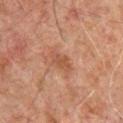  biopsy_status: not biopsied; imaged during a skin examination
  image:
    source: total-body photography crop
    field_of_view_mm: 15
  lesion_size:
    long_diameter_mm_approx: 3.0
  patient:
    sex: male
    age_approx: 60
  site: chest
  lighting: cross-polarized